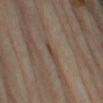notes = no biopsy performed (imaged during a skin exam) | lesion diameter = ≈2.5 mm | image = 15 mm crop, total-body photography | patient = female, aged 63 to 67 | TBP lesion metrics = a lesion color around L≈41 a*≈13 b*≈22 in CIELAB, roughly 6 lightness units darker than nearby skin, and a normalized lesion–skin contrast near 5.5; internal color variation of about 0 on a 0–10 scale and peripheral color asymmetry of about 0 | site = the left upper arm | lighting = cross-polarized illumination.